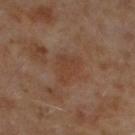  biopsy_status: not biopsied; imaged during a skin examination
  site: left lower leg
  patient:
    sex: male
    age_approx: 60
  automated_metrics:
    eccentricity: 0.75
    shape_asymmetry: 0.4
    cielab_L: 36
    cielab_a: 18
    cielab_b: 27
    vs_skin_contrast_norm: 5.0
    border_irregularity_0_10: 4.0
    color_variation_0_10: 1.5
    peripheral_color_asymmetry: 0.5
  image:
    source: total-body photography crop
    field_of_view_mm: 15
  lesion_size:
    long_diameter_mm_approx: 4.0
  lighting: cross-polarized